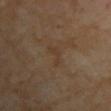No biopsy was performed on this lesion — it was imaged during a full skin examination and was not determined to be concerning.
A female subject approximately 60 years of age.
The lesion is located on the chest.
Automated image analysis of the tile measured an average lesion color of about L≈36 a*≈14 b*≈28 (CIELAB), a lesion–skin lightness drop of about 5, and a normalized border contrast of about 5.5. It also reported an automated nevus-likeness rating near 0 out of 100 and a lesion-detection confidence of about 100/100.
The recorded lesion diameter is about 3 mm.
A 15 mm close-up extracted from a 3D total-body photography capture.